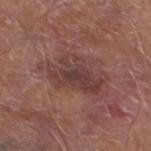workup: no biopsy performed (imaged during a skin exam) | lesion size: ~6 mm (longest diameter) | image: ~15 mm crop, total-body skin-cancer survey | TBP lesion metrics: roughly 8 lightness units darker than nearby skin and a lesion-to-skin contrast of about 7 (normalized; higher = more distinct); a within-lesion color-variation index near 3.5/10 and a peripheral color-asymmetry measure near 1; a lesion-detection confidence of about 100/100 | site: the left lower leg | lighting: white-light illumination | subject: male, aged around 65.